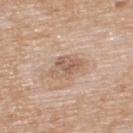Image and clinical context: The lesion is located on the back. A roughly 15 mm field-of-view crop from a total-body skin photograph. A male patient, aged approximately 60.